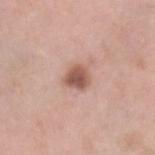• notes — no biopsy performed (imaged during a skin exam)
• acquisition — total-body-photography crop, ~15 mm field of view
• automated metrics — a lesion area of about 5 mm² and a symmetry-axis asymmetry near 0.25; an average lesion color of about L≈54 a*≈22 b*≈27 (CIELAB), a lesion–skin lightness drop of about 14, and a normalized lesion–skin contrast near 9.5; border irregularity of about 2 on a 0–10 scale and radial color variation of about 1; lesion-presence confidence of about 100/100
• subject — female, approximately 40 years of age
• location — the leg
• lighting — white-light illumination